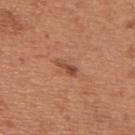– workup — no biopsy performed (imaged during a skin exam)
– body site — the upper back
– image-analysis metrics — a lesion area of about 2.5 mm² and an eccentricity of roughly 0.9; an automated nevus-likeness rating near 20 out of 100 and a detector confidence of about 100 out of 100 that the crop contains a lesion
– patient — male, aged around 65
– tile lighting — white-light illumination
– diameter — about 2.5 mm
– image — ~15 mm tile from a whole-body skin photo